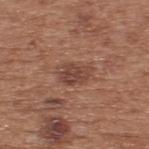Context: Located on the back. This image is a 15 mm lesion crop taken from a total-body photograph. The patient is a male about 55 years old.